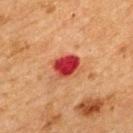workup — no biopsy performed (imaged during a skin exam)